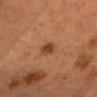Imaged during a routine full-body skin examination; the lesion was not biopsied and no histopathology is available. Approximately 3 mm at its widest. Located on the head or neck. A roughly 15 mm field-of-view crop from a total-body skin photograph. The subject is a female roughly 40 years of age. Captured under cross-polarized illumination.